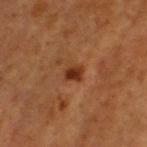The recorded lesion diameter is about 2 mm. This image is a 15 mm lesion crop taken from a total-body photograph. An algorithmic analysis of the crop reported a lesion color around L≈32 a*≈25 b*≈32 in CIELAB, about 12 CIELAB-L* units darker than the surrounding skin, and a lesion-to-skin contrast of about 10.5 (normalized; higher = more distinct). The software also gave a border-irregularity index near 2/10, a within-lesion color-variation index near 2/10, and radial color variation of about 0.5. The software also gave a nevus-likeness score of about 90/100 and lesion-presence confidence of about 100/100. This is a cross-polarized tile. The lesion is on the head or neck. A male patient aged 58 to 62.